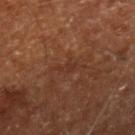Q: Was a biopsy performed?
A: no biopsy performed (imaged during a skin exam)
Q: What are the patient's age and sex?
A: aged 63 to 67
Q: What is the imaging modality?
A: 15 mm crop, total-body photography
Q: Lesion location?
A: the right lower leg
Q: How large is the lesion?
A: ~2.5 mm (longest diameter)
Q: How was the tile lit?
A: cross-polarized